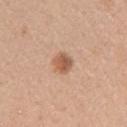Recorded during total-body skin imaging; not selected for excision or biopsy. Located on the left upper arm. Captured under white-light illumination. A female patient, in their 30s. Cropped from a whole-body photographic skin survey; the tile spans about 15 mm. Measured at roughly 2.5 mm in maximum diameter.